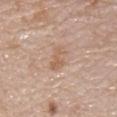This lesion was catalogued during total-body skin photography and was not selected for biopsy. Imaged with white-light lighting. A region of skin cropped from a whole-body photographic capture, roughly 15 mm wide. A female subject, in their mid- to late 40s. Automated tile analysis of the lesion measured a detector confidence of about 100 out of 100 that the crop contains a lesion. Approximately 3.5 mm at its widest. On the chest.